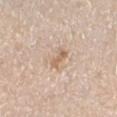Clinical impression:
This lesion was catalogued during total-body skin photography and was not selected for biopsy.
Context:
A female patient, roughly 45 years of age. The tile uses white-light illumination. Measured at roughly 3 mm in maximum diameter. A lesion tile, about 15 mm wide, cut from a 3D total-body photograph. An algorithmic analysis of the crop reported an area of roughly 3 mm², an outline eccentricity of about 0.95 (0 = round, 1 = elongated), and a shape-asymmetry score of about 0.3 (0 = symmetric). The software also gave a lesion color around L≈63 a*≈16 b*≈31 in CIELAB, a lesion–skin lightness drop of about 10, and a normalized lesion–skin contrast near 6.5. The software also gave a border-irregularity index near 4/10, a color-variation rating of about 0/10, and peripheral color asymmetry of about 0. And it measured a nevus-likeness score of about 20/100. The lesion is on the right forearm.